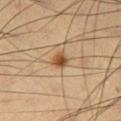Assessment: This lesion was catalogued during total-body skin photography and was not selected for biopsy.